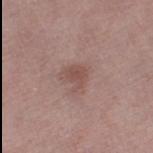The lesion was tiled from a total-body skin photograph and was not biopsied.
About 2.5 mm across.
On the left thigh.
The tile uses white-light illumination.
Automated image analysis of the tile measured a lesion area of about 4.5 mm² and an eccentricity of roughly 0.55. It also reported border irregularity of about 3.5 on a 0–10 scale, internal color variation of about 2 on a 0–10 scale, and a peripheral color-asymmetry measure near 0.5. It also reported a nevus-likeness score of about 20/100 and a lesion-detection confidence of about 100/100.
A female patient aged approximately 70.
Cropped from a total-body skin-imaging series; the visible field is about 15 mm.